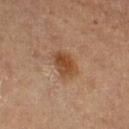Longest diameter approximately 3.5 mm. This is a cross-polarized tile. Located on the right thigh. A 15 mm crop from a total-body photograph taken for skin-cancer surveillance. A male subject in their mid- to late 80s or older. An algorithmic analysis of the crop reported an area of roughly 7 mm², a shape eccentricity near 0.8, and two-axis asymmetry of about 0.2. And it measured a border-irregularity rating of about 2/10 and peripheral color asymmetry of about 1.5. It also reported a nevus-likeness score of about 90/100 and a lesion-detection confidence of about 100/100.